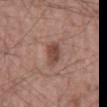biopsy status: no biopsy performed (imaged during a skin exam)
subject: male, aged around 55
size: ~3 mm (longest diameter)
automated metrics: a shape eccentricity near 0.75 and two-axis asymmetry of about 0.2; a border-irregularity index near 2/10, internal color variation of about 2.5 on a 0–10 scale, and a peripheral color-asymmetry measure near 1
tile lighting: white-light illumination
imaging modality: total-body-photography crop, ~15 mm field of view
site: the mid back